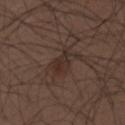No biopsy was performed on this lesion — it was imaged during a full skin examination and was not determined to be concerning. A lesion tile, about 15 mm wide, cut from a 3D total-body photograph. The lesion is on the upper back. The lesion-visualizer software estimated a border-irregularity rating of about 5.5/10, internal color variation of about 2.5 on a 0–10 scale, and peripheral color asymmetry of about 0.5. Longest diameter approximately 4 mm. Captured under white-light illumination. The patient is a male about 30 years old.